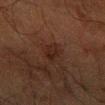Impression: Recorded during total-body skin imaging; not selected for excision or biopsy. Acquisition and patient details: A male subject aged 58–62. The lesion-visualizer software estimated a symmetry-axis asymmetry near 0.3. Captured under cross-polarized illumination. On the right forearm. Cropped from a total-body skin-imaging series; the visible field is about 15 mm.